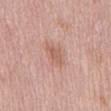No biopsy was performed on this lesion — it was imaged during a full skin examination and was not determined to be concerning.
A 15 mm crop from a total-body photograph taken for skin-cancer surveillance.
The lesion is on the mid back.
A female patient approximately 60 years of age.